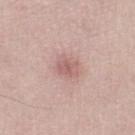Impression:
The lesion was photographed on a routine skin check and not biopsied; there is no pathology result.
Background:
The total-body-photography lesion software estimated an area of roughly 5 mm² and an eccentricity of roughly 0.75. And it measured an average lesion color of about L≈60 a*≈21 b*≈22 (CIELAB), roughly 9 lightness units darker than nearby skin, and a lesion-to-skin contrast of about 6 (normalized; higher = more distinct). And it measured border irregularity of about 2 on a 0–10 scale, a within-lesion color-variation index near 2/10, and radial color variation of about 0.5. A 15 mm close-up tile from a total-body photography series done for melanoma screening. The patient is a male about 60 years old. The lesion's longest dimension is about 3 mm. The lesion is located on the right lower leg.